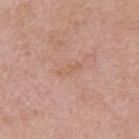workup=catalogued during a skin exam; not biopsied | illumination=white-light | TBP lesion metrics=a lesion-detection confidence of about 100/100 | diameter=~3 mm (longest diameter) | image=15 mm crop, total-body photography | subject=male, approximately 60 years of age | site=the back.